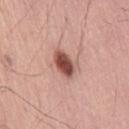Image and clinical context: The recorded lesion diameter is about 3.5 mm. From the lower back. An algorithmic analysis of the crop reported a lesion area of about 7.5 mm², an outline eccentricity of about 0.75 (0 = round, 1 = elongated), and two-axis asymmetry of about 0.25. The software also gave a border-irregularity rating of about 2.5/10, a color-variation rating of about 5.5/10, and a peripheral color-asymmetry measure near 1.5. It also reported a nevus-likeness score of about 100/100 and a lesion-detection confidence of about 100/100. The tile uses white-light illumination. A close-up tile cropped from a whole-body skin photograph, about 15 mm across. The patient is a male in their 60s.